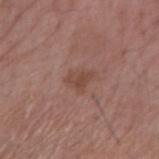No biopsy was performed on this lesion — it was imaged during a full skin examination and was not determined to be concerning.
A male patient, aged 53–57.
A close-up tile cropped from a whole-body skin photograph, about 15 mm across.
The lesion is located on the arm.
Approximately 3 mm at its widest.
Automated image analysis of the tile measured a shape eccentricity near 0.65 and a shape-asymmetry score of about 0.45 (0 = symmetric). The analysis additionally found an automated nevus-likeness rating near 5 out of 100 and a detector confidence of about 100 out of 100 that the crop contains a lesion.